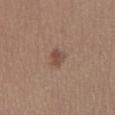follow-up: no biopsy performed (imaged during a skin exam) | body site: the right lower leg | tile lighting: white-light | diameter: about 2.5 mm | automated lesion analysis: an average lesion color of about L≈48 a*≈18 b*≈26 (CIELAB), about 9 CIELAB-L* units darker than the surrounding skin, and a lesion-to-skin contrast of about 7 (normalized; higher = more distinct); border irregularity of about 2.5 on a 0–10 scale, a within-lesion color-variation index near 2/10, and radial color variation of about 0.5 | patient: female, aged around 40 | acquisition: ~15 mm crop, total-body skin-cancer survey.